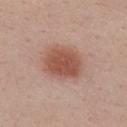{
  "biopsy_status": "not biopsied; imaged during a skin examination",
  "lighting": "white-light",
  "site": "mid back",
  "image": {
    "source": "total-body photography crop",
    "field_of_view_mm": 15
  },
  "lesion_size": {
    "long_diameter_mm_approx": 5.0
  },
  "patient": {
    "sex": "male",
    "age_approx": 40
  }
}